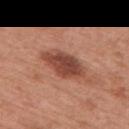Q: Is there a histopathology result?
A: total-body-photography surveillance lesion; no biopsy
Q: What is the anatomic site?
A: the upper back
Q: What are the patient's age and sex?
A: female, aged 48 to 52
Q: What kind of image is this?
A: ~15 mm tile from a whole-body skin photo